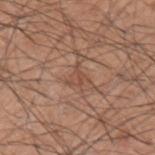Imaged during a routine full-body skin examination; the lesion was not biopsied and no histopathology is available. The lesion is on the left upper arm. About 2.5 mm across. A male subject approximately 60 years of age. A 15 mm close-up extracted from a 3D total-body photography capture.